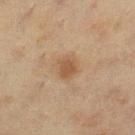Part of a total-body skin-imaging series; this lesion was reviewed on a skin check and was not flagged for biopsy. The patient is a female in their mid-50s. The lesion is located on the left lower leg. A 15 mm close-up extracted from a 3D total-body photography capture.